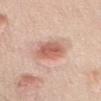Impression: Part of a total-body skin-imaging series; this lesion was reviewed on a skin check and was not flagged for biopsy. Acquisition and patient details: A 15 mm crop from a total-body photograph taken for skin-cancer surveillance. A male patient in their 50s. The lesion is on the chest. Captured under white-light illumination. Approximately 4 mm at its widest.